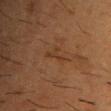Captured under cross-polarized illumination. A roughly 15 mm field-of-view crop from a total-body skin photograph. The patient is a male in their mid- to late 50s. An algorithmic analysis of the crop reported a footprint of about 3 mm², an outline eccentricity of about 0.85 (0 = round, 1 = elongated), and a shape-asymmetry score of about 0.7 (0 = symmetric). The software also gave an average lesion color of about L≈31 a*≈17 b*≈28 (CIELAB), roughly 5 lightness units darker than nearby skin, and a normalized lesion–skin contrast near 5. The software also gave an automated nevus-likeness rating near 0 out of 100 and lesion-presence confidence of about 70/100. From the upper back.